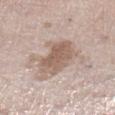Q: Is there a histopathology result?
A: no biopsy performed (imaged during a skin exam)
Q: What are the patient's age and sex?
A: female, aged 38 to 42
Q: Where on the body is the lesion?
A: the left lower leg
Q: How was this image acquired?
A: total-body-photography crop, ~15 mm field of view